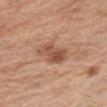Case summary:
* patient: male, aged 68 to 72
* acquisition: ~15 mm crop, total-body skin-cancer survey
* size: ≈3.5 mm
* TBP lesion metrics: a footprint of about 8 mm² and a shape-asymmetry score of about 0.2 (0 = symmetric); a border-irregularity index near 2.5/10, a within-lesion color-variation index near 5/10, and peripheral color asymmetry of about 2; a nevus-likeness score of about 35/100
* tile lighting: white-light
* anatomic site: the arm